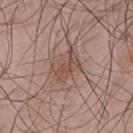Q: Was a biopsy performed?
A: no biopsy performed (imaged during a skin exam)
Q: How was this image acquired?
A: ~15 mm tile from a whole-body skin photo
Q: Automated lesion metrics?
A: a shape eccentricity near 0.7 and a shape-asymmetry score of about 0.3 (0 = symmetric); a border-irregularity index near 3.5/10, a within-lesion color-variation index near 4/10, and a peripheral color-asymmetry measure near 1.5
Q: Lesion size?
A: ≈4 mm
Q: What are the patient's age and sex?
A: male, aged around 45
Q: Where on the body is the lesion?
A: the upper back
Q: What lighting was used for the tile?
A: white-light